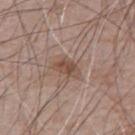Q: Was a biopsy performed?
A: total-body-photography surveillance lesion; no biopsy